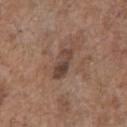No biopsy was performed on this lesion — it was imaged during a full skin examination and was not determined to be concerning. This is a white-light tile. A region of skin cropped from a whole-body photographic capture, roughly 15 mm wide. A male patient aged 68 to 72. The lesion is on the front of the torso. Measured at roughly 4 mm in maximum diameter.